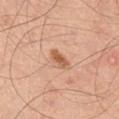Background: A roughly 15 mm field-of-view crop from a total-body skin photograph. The patient is a male approximately 45 years of age. Imaged with white-light lighting. An algorithmic analysis of the crop reported a footprint of about 4 mm², an outline eccentricity of about 0.9 (0 = round, 1 = elongated), and two-axis asymmetry of about 0.2. The software also gave an average lesion color of about L≈60 a*≈24 b*≈35 (CIELAB), about 11 CIELAB-L* units darker than the surrounding skin, and a lesion-to-skin contrast of about 7.5 (normalized; higher = more distinct). It also reported a color-variation rating of about 2.5/10. And it measured a detector confidence of about 100 out of 100 that the crop contains a lesion. The lesion is on the chest. The recorded lesion diameter is about 3 mm.